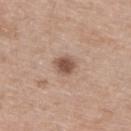Captured during whole-body skin photography for melanoma surveillance; the lesion was not biopsied.
Automated tile analysis of the lesion measured a mean CIELAB color near L≈52 a*≈19 b*≈27 and roughly 13 lightness units darker than nearby skin.
Located on the upper back.
Cropped from a whole-body photographic skin survey; the tile spans about 15 mm.
Captured under white-light illumination.
The subject is a male aged 38–42.